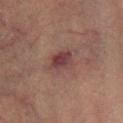- notes — imaged on a skin check; not biopsied
- lesion diameter — about 3.5 mm
- patient — male, in their mid-50s
- site — the left lower leg
- tile lighting — cross-polarized
- imaging modality — 15 mm crop, total-body photography